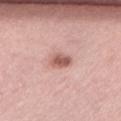workup = imaged on a skin check; not biopsied | subject = female, roughly 50 years of age | acquisition = 15 mm crop, total-body photography | tile lighting = white-light | location = the lower back | diameter = about 2.5 mm.